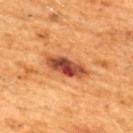Histopathology of the biopsied lesion showed a melanoma in situ arising in association with a nevus (malignant).